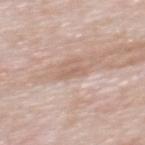Case summary:
• notes: imaged on a skin check; not biopsied
• imaging modality: ~15 mm tile from a whole-body skin photo
• subject: male, approximately 55 years of age
• lighting: white-light illumination
• body site: the mid back
• lesion diameter: ≈2.5 mm